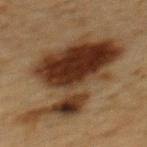A male patient aged approximately 60. Automated image analysis of the tile measured a border-irregularity index near 7.5/10 and a within-lesion color-variation index near 8.5/10. Cropped from a whole-body photographic skin survey; the tile spans about 15 mm. Captured under cross-polarized illumination. About 12.5 mm across. On the mid back.